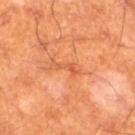body site: the leg | illumination: cross-polarized | acquisition: ~15 mm crop, total-body skin-cancer survey | lesion diameter: ~2.5 mm (longest diameter) | subject: male, aged around 60.